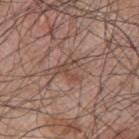A male subject, about 50 years old.
Automated tile analysis of the lesion measured a footprint of about 4 mm², a shape eccentricity near 0.55, and a symmetry-axis asymmetry near 0.7. The analysis additionally found a lesion color around L≈47 a*≈18 b*≈25 in CIELAB and about 7 CIELAB-L* units darker than the surrounding skin.
Located on the upper back.
Captured under white-light illumination.
A 15 mm crop from a total-body photograph taken for skin-cancer surveillance.